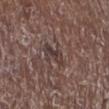Findings:
- notes — no biopsy performed (imaged during a skin exam)
- illumination — white-light
- TBP lesion metrics — an area of roughly 4.5 mm², a shape eccentricity near 0.9, and two-axis asymmetry of about 0.4
- subject — male, aged approximately 70
- diameter — ~3.5 mm (longest diameter)
- anatomic site — the right lower leg
- image — 15 mm crop, total-body photography